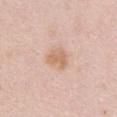{"biopsy_status": "not biopsied; imaged during a skin examination", "image": {"source": "total-body photography crop", "field_of_view_mm": 15}, "lesion_size": {"long_diameter_mm_approx": 3.0}, "lighting": "white-light", "site": "chest", "patient": {"sex": "female", "age_approx": 40}}